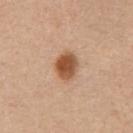body site: the chest
subject: male, aged approximately 50
tile lighting: white-light
acquisition: ~15 mm tile from a whole-body skin photo